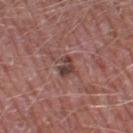Assessment:
Imaged during a routine full-body skin examination; the lesion was not biopsied and no histopathology is available.
Background:
The lesion is on the right thigh. Measured at roughly 3 mm in maximum diameter. Cropped from a total-body skin-imaging series; the visible field is about 15 mm. The patient is a male about 60 years old.